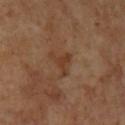A 15 mm close-up tile from a total-body photography series done for melanoma screening. Located on the left lower leg. Longest diameter approximately 3.5 mm. The total-body-photography lesion software estimated a footprint of about 4.5 mm², an outline eccentricity of about 0.6 (0 = round, 1 = elongated), and two-axis asymmetry of about 0.7. The software also gave radial color variation of about 0.5. And it measured a nevus-likeness score of about 0/100. The patient is a female aged 48 to 52. The tile uses cross-polarized illumination.